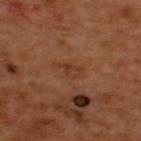{
  "biopsy_status": "not biopsied; imaged during a skin examination"
}